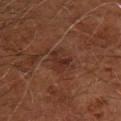Case summary:
* size — ≈3.5 mm
* image — ~15 mm crop, total-body skin-cancer survey
* subject — male, aged 63–67
* automated metrics — an area of roughly 6 mm² and an outline eccentricity of about 0.7 (0 = round, 1 = elongated)